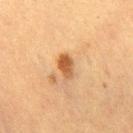Imaged during a routine full-body skin examination; the lesion was not biopsied and no histopathology is available. Automated tile analysis of the lesion measured an average lesion color of about L≈47 a*≈21 b*≈35 (CIELAB) and a normalized border contrast of about 9. The software also gave a within-lesion color-variation index near 4/10 and radial color variation of about 1.5. And it measured an automated nevus-likeness rating near 95 out of 100. The lesion is located on the leg. The subject is a female aged 53–57. This image is a 15 mm lesion crop taken from a total-body photograph. Measured at roughly 3 mm in maximum diameter.